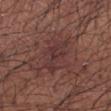notes: catalogued during a skin exam; not biopsied
illumination: white-light illumination
patient: male, in their mid-50s
TBP lesion metrics: an average lesion color of about L≈34 a*≈20 b*≈19 (CIELAB) and a lesion–skin lightness drop of about 5
imaging modality: ~15 mm tile from a whole-body skin photo
anatomic site: the left forearm